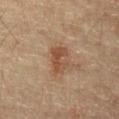- notes — total-body-photography surveillance lesion; no biopsy
- illumination — cross-polarized illumination
- imaging modality — ~15 mm tile from a whole-body skin photo
- patient — male, roughly 60 years of age